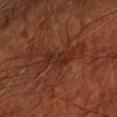Recorded during total-body skin imaging; not selected for excision or biopsy. A 15 mm close-up extracted from a 3D total-body photography capture. On the left forearm. The lesion's longest dimension is about 4.5 mm. The patient is aged around 65. Imaged with cross-polarized lighting.